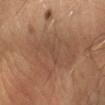Q: Was this lesion biopsied?
A: catalogued during a skin exam; not biopsied
Q: Who is the patient?
A: male, aged approximately 40
Q: Automated lesion metrics?
A: a lesion color around L≈46 a*≈18 b*≈28 in CIELAB, a lesion–skin lightness drop of about 4, and a lesion-to-skin contrast of about 3 (normalized; higher = more distinct); a color-variation rating of about 0/10; an automated nevus-likeness rating near 0 out of 100 and lesion-presence confidence of about 95/100
Q: What lighting was used for the tile?
A: cross-polarized illumination
Q: What is the lesion's diameter?
A: ~2.5 mm (longest diameter)
Q: How was this image acquired?
A: ~15 mm tile from a whole-body skin photo
Q: What is the anatomic site?
A: the arm